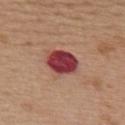| field | value |
|---|---|
| biopsy status | total-body-photography surveillance lesion; no biopsy |
| automated lesion analysis | a lesion area of about 10 mm², an eccentricity of roughly 0.6, and two-axis asymmetry of about 0.2; an automated nevus-likeness rating near 0 out of 100 and a lesion-detection confidence of about 100/100 |
| lesion diameter | about 4 mm |
| location | the upper back |
| image | 15 mm crop, total-body photography |
| lighting | white-light illumination |
| patient | female, about 40 years old |